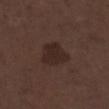Assessment:
This lesion was catalogued during total-body skin photography and was not selected for biopsy.
Acquisition and patient details:
From the right lower leg. Cropped from a whole-body photographic skin survey; the tile spans about 15 mm. Longest diameter approximately 4 mm. Automated tile analysis of the lesion measured a mean CIELAB color near L≈25 a*≈14 b*≈19 and about 7 CIELAB-L* units darker than the surrounding skin. Imaged with white-light lighting. A male subject, about 70 years old.